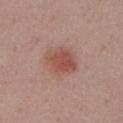biopsy status = imaged on a skin check; not biopsied | imaging modality = ~15 mm crop, total-body skin-cancer survey | site = the chest | patient = female, roughly 55 years of age | lesion diameter = about 3.5 mm | tile lighting = white-light.